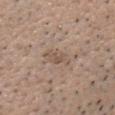Impression: Captured during whole-body skin photography for melanoma surveillance; the lesion was not biopsied. Acquisition and patient details: A male subject, aged 48 to 52. A lesion tile, about 15 mm wide, cut from a 3D total-body photograph. From the head or neck.